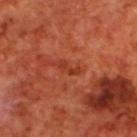Imaged during a routine full-body skin examination; the lesion was not biopsied and no histopathology is available. The patient is a male roughly 70 years of age. Imaged with cross-polarized lighting. Approximately 3 mm at its widest. The lesion-visualizer software estimated a lesion color around L≈38 a*≈33 b*≈36 in CIELAB and a normalized lesion–skin contrast near 6. The software also gave a border-irregularity rating of about 5/10, internal color variation of about 0 on a 0–10 scale, and radial color variation of about 0. A lesion tile, about 15 mm wide, cut from a 3D total-body photograph. Located on the upper back.